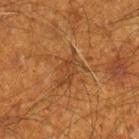Part of a total-body skin-imaging series; this lesion was reviewed on a skin check and was not flagged for biopsy.
The lesion is located on the right lower leg.
The lesion-visualizer software estimated a footprint of about 7.5 mm², a shape eccentricity near 0.8, and a symmetry-axis asymmetry near 0.45. The analysis additionally found a mean CIELAB color near L≈36 a*≈20 b*≈33, roughly 6 lightness units darker than nearby skin, and a normalized border contrast of about 5.5. The software also gave a nevus-likeness score of about 0/100.
A male patient, aged around 60.
This is a cross-polarized tile.
Cropped from a total-body skin-imaging series; the visible field is about 15 mm.
Measured at roughly 4.5 mm in maximum diameter.